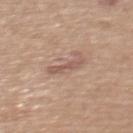This lesion was catalogued during total-body skin photography and was not selected for biopsy. On the upper back. The lesion's longest dimension is about 3.5 mm. This is a white-light tile. Cropped from a total-body skin-imaging series; the visible field is about 15 mm. Automated tile analysis of the lesion measured an outline eccentricity of about 0.9 (0 = round, 1 = elongated). It also reported an average lesion color of about L≈55 a*≈19 b*≈26 (CIELAB), about 9 CIELAB-L* units darker than the surrounding skin, and a normalized lesion–skin contrast near 6. The subject is a female aged 53 to 57.